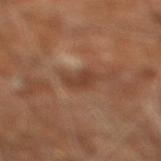No biopsy was performed on this lesion — it was imaged during a full skin examination and was not determined to be concerning. Imaged with cross-polarized lighting. A region of skin cropped from a whole-body photographic capture, roughly 15 mm wide. From the right leg. A male subject, aged approximately 60. Measured at roughly 3 mm in maximum diameter.